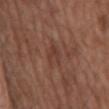* biopsy status — imaged on a skin check; not biopsied
* imaging modality — ~15 mm tile from a whole-body skin photo
* diameter — about 3.5 mm
* automated lesion analysis — a footprint of about 5.5 mm²; a border-irregularity index near 3/10 and radial color variation of about 1; a classifier nevus-likeness of about 0/100 and a detector confidence of about 85 out of 100 that the crop contains a lesion
* site — the back
* subject — male, aged around 75